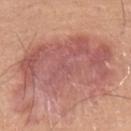Q: Was a biopsy performed?
A: catalogued during a skin exam; not biopsied
Q: Lesion location?
A: the left lower leg
Q: Who is the patient?
A: female, in their mid- to late 40s
Q: What is the imaging modality?
A: ~15 mm tile from a whole-body skin photo